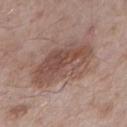notes: no biopsy performed (imaged during a skin exam)
illumination: white-light
body site: the right upper arm
image-analysis metrics: an automated nevus-likeness rating near 35 out of 100 and a detector confidence of about 100 out of 100 that the crop contains a lesion
diameter: about 7 mm
patient: male, aged 53 to 57
imaging modality: ~15 mm tile from a whole-body skin photo